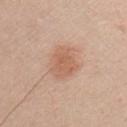| feature | finding |
|---|---|
| notes | catalogued during a skin exam; not biopsied |
| anatomic site | the chest |
| patient | male, aged approximately 30 |
| automated metrics | a shape eccentricity near 0.7 and a shape-asymmetry score of about 0.25 (0 = symmetric); an average lesion color of about L≈61 a*≈21 b*≈31 (CIELAB), a lesion–skin lightness drop of about 8, and a normalized border contrast of about 6 |
| acquisition | ~15 mm crop, total-body skin-cancer survey |
| lesion diameter | ≈4.5 mm |